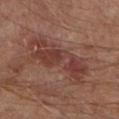Q: Was this lesion biopsied?
A: no biopsy performed (imaged during a skin exam)
Q: What is the imaging modality?
A: total-body-photography crop, ~15 mm field of view
Q: What are the patient's age and sex?
A: male, roughly 65 years of age
Q: Where on the body is the lesion?
A: the left lower leg
Q: What lighting was used for the tile?
A: cross-polarized